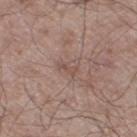notes — no biopsy performed (imaged during a skin exam); tile lighting — white-light illumination; diameter — about 2.5 mm; TBP lesion metrics — an area of roughly 2 mm², an outline eccentricity of about 0.9 (0 = round, 1 = elongated), and a shape-asymmetry score of about 0.45 (0 = symmetric); subject — male, roughly 55 years of age; imaging modality — ~15 mm crop, total-body skin-cancer survey; body site — the leg.